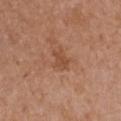Findings:
• workup · no biopsy performed (imaged during a skin exam)
• lesion size · ≈2.5 mm
• image-analysis metrics · an area of roughly 4 mm², an outline eccentricity of about 0.6 (0 = round, 1 = elongated), and a symmetry-axis asymmetry near 0.35; an average lesion color of about L≈49 a*≈23 b*≈33 (CIELAB), a lesion–skin lightness drop of about 7, and a normalized border contrast of about 5.5; a border-irregularity rating of about 3.5/10; a nevus-likeness score of about 0/100 and a detector confidence of about 100 out of 100 that the crop contains a lesion
• subject · female, aged approximately 65
• tile lighting · white-light
• location · the front of the torso
• acquisition · 15 mm crop, total-body photography